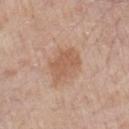{"biopsy_status": "not biopsied; imaged during a skin examination", "image": {"source": "total-body photography crop", "field_of_view_mm": 15}, "patient": {"sex": "male", "age_approx": 80}, "lighting": "white-light", "site": "chest"}